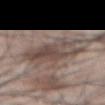The subject is a male aged 43–47. Approximately 7 mm at its widest. A 15 mm crop from a total-body photograph taken for skin-cancer surveillance. From the abdomen. An algorithmic analysis of the crop reported an area of roughly 20 mm² and an outline eccentricity of about 0.85 (0 = round, 1 = elongated). And it measured a mean CIELAB color near L≈47 a*≈13 b*≈20 and a normalized border contrast of about 8. It also reported radial color variation of about 2.